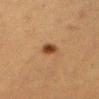Q: Was a biopsy performed?
A: no biopsy performed (imaged during a skin exam)
Q: Lesion size?
A: about 2.5 mm
Q: Illumination type?
A: cross-polarized
Q: Lesion location?
A: the right thigh
Q: Patient demographics?
A: female, aged approximately 55
Q: What is the imaging modality?
A: 15 mm crop, total-body photography